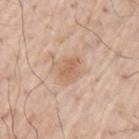Clinical impression:
Recorded during total-body skin imaging; not selected for excision or biopsy.
Background:
Automated image analysis of the tile measured a lesion area of about 4.5 mm², an eccentricity of roughly 0.75, and two-axis asymmetry of about 0.3. And it measured roughly 8 lightness units darker than nearby skin and a normalized lesion–skin contrast near 6. Imaged with white-light lighting. From the left upper arm. Measured at roughly 3 mm in maximum diameter. A region of skin cropped from a whole-body photographic capture, roughly 15 mm wide. A male subject about 70 years old.